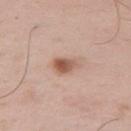Recorded during total-body skin imaging; not selected for excision or biopsy. About 3 mm across. The total-body-photography lesion software estimated a lesion area of about 5 mm², an eccentricity of roughly 0.65, and a shape-asymmetry score of about 0.25 (0 = symmetric). It also reported a border-irregularity index near 2.5/10, a color-variation rating of about 6/10, and peripheral color asymmetry of about 2. The software also gave a nevus-likeness score of about 95/100 and lesion-presence confidence of about 100/100. Captured under white-light illumination. A 15 mm close-up extracted from a 3D total-body photography capture. From the right upper arm. A male patient in their mid-50s.